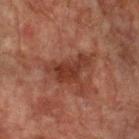No biopsy was performed on this lesion — it was imaged during a full skin examination and was not determined to be concerning.
An algorithmic analysis of the crop reported an outline eccentricity of about 0.85 (0 = round, 1 = elongated) and a shape-asymmetry score of about 0.5 (0 = symmetric). The software also gave a lesion color around L≈30 a*≈22 b*≈24 in CIELAB, roughly 8 lightness units darker than nearby skin, and a normalized lesion–skin contrast near 8.
A close-up tile cropped from a whole-body skin photograph, about 15 mm across.
A male patient in their mid-70s.
Longest diameter approximately 5 mm.
This is a cross-polarized tile.
The lesion is located on the left forearm.